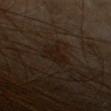| key | value |
|---|---|
| follow-up | total-body-photography surveillance lesion; no biopsy |
| automated metrics | a lesion color around L≈16 a*≈11 b*≈19 in CIELAB, about 5 CIELAB-L* units darker than the surrounding skin, and a lesion-to-skin contrast of about 7 (normalized; higher = more distinct); a border-irregularity index near 5/10, a within-lesion color-variation index near 2/10, and peripheral color asymmetry of about 0.5; a classifier nevus-likeness of about 0/100 |
| location | the head or neck |
| image | 15 mm crop, total-body photography |
| lesion diameter | ~4 mm (longest diameter) |
| patient | female, about 70 years old |